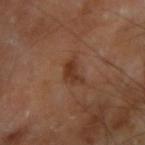<case>
<biopsy_status>not biopsied; imaged during a skin examination</biopsy_status>
<image>
  <source>total-body photography crop</source>
  <field_of_view_mm>15</field_of_view_mm>
</image>
<patient>
  <sex>male</sex>
  <age_approx>65</age_approx>
</patient>
<site>right upper arm</site>
</case>